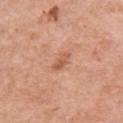Notes:
- biopsy status — catalogued during a skin exam; not biopsied
- acquisition — 15 mm crop, total-body photography
- site — the right upper arm
- automated lesion analysis — a lesion color around L≈58 a*≈26 b*≈34 in CIELAB and a lesion-to-skin contrast of about 6.5 (normalized; higher = more distinct)
- patient — female, about 40 years old